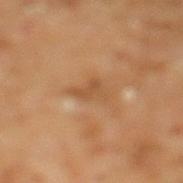{"biopsy_status": "not biopsied; imaged during a skin examination", "patient": {"sex": "male", "age_approx": 60}, "site": "leg", "image": {"source": "total-body photography crop", "field_of_view_mm": 15}}